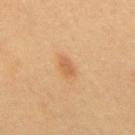Notes:
- biopsy status · catalogued during a skin exam; not biopsied
- patient · female, about 50 years old
- illumination · cross-polarized illumination
- location · the mid back
- acquisition · total-body-photography crop, ~15 mm field of view